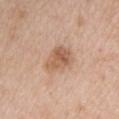<lesion>
<biopsy_status>not biopsied; imaged during a skin examination</biopsy_status>
<image>
  <source>total-body photography crop</source>
  <field_of_view_mm>15</field_of_view_mm>
</image>
<automated_metrics>
  <cielab_L>59</cielab_L>
  <cielab_a>20</cielab_a>
  <cielab_b>32</cielab_b>
  <vs_skin_darker_L>10.0</vs_skin_darker_L>
</automated_metrics>
<lighting>white-light</lighting>
<site>upper back</site>
<patient>
  <sex>female</sex>
  <age_approx>45</age_approx>
</patient>
</lesion>